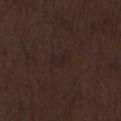follow-up = imaged on a skin check; not biopsied
patient = male, aged around 70
anatomic site = the leg
acquisition = ~15 mm crop, total-body skin-cancer survey
lesion diameter = ≈2.5 mm